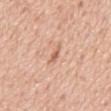follow-up=no biopsy performed (imaged during a skin exam) | location=the mid back | imaging modality=15 mm crop, total-body photography | subject=male, in their 60s.